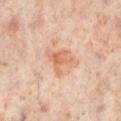workup: total-body-photography surveillance lesion; no biopsy
subject: female, aged 48–52
tile lighting: cross-polarized
location: the right lower leg
image source: ~15 mm crop, total-body skin-cancer survey